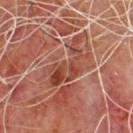Part of a total-body skin-imaging series; this lesion was reviewed on a skin check and was not flagged for biopsy. Measured at roughly 6.5 mm in maximum diameter. A male subject, aged 58–62. The total-body-photography lesion software estimated a footprint of about 12 mm², an eccentricity of roughly 0.85, and a symmetry-axis asymmetry near 0.65. Located on the upper back. Captured under cross-polarized illumination. Cropped from a total-body skin-imaging series; the visible field is about 15 mm.